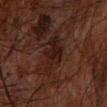• illumination: cross-polarized illumination
• patient: male, in their mid- to late 60s
• image source: ~15 mm crop, total-body skin-cancer survey
• site: the left forearm
• image-analysis metrics: an average lesion color of about L≈15 a*≈15 b*≈16 (CIELAB) and a normalized lesion–skin contrast near 8; border irregularity of about 4.5 on a 0–10 scale, a color-variation rating of about 2.5/10, and peripheral color asymmetry of about 1; an automated nevus-likeness rating near 0 out of 100 and lesion-presence confidence of about 75/100
• lesion diameter: ~5.5 mm (longest diameter)